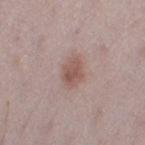<case>
<biopsy_status>not biopsied; imaged during a skin examination</biopsy_status>
<image>
  <source>total-body photography crop</source>
  <field_of_view_mm>15</field_of_view_mm>
</image>
<lighting>white-light</lighting>
<patient>
  <sex>female</sex>
  <age_approx>30</age_approx>
</patient>
<site>left thigh</site>
</case>